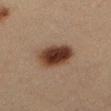Notes:
– follow-up · catalogued during a skin exam; not biopsied
– location · the leg
– acquisition · total-body-photography crop, ~15 mm field of view
– patient · female, aged 28 to 32